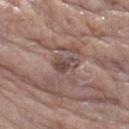biopsy_status: not biopsied; imaged during a skin examination
automated_metrics:
  area_mm2_approx: 4.5
  eccentricity: 0.75
  shape_asymmetry: 0.35
lesion_size:
  long_diameter_mm_approx: 3.0
site: right thigh
lighting: white-light
patient:
  sex: female
  age_approx: 85+
image:
  source: total-body photography crop
  field_of_view_mm: 15